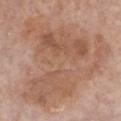Part of a total-body skin-imaging series; this lesion was reviewed on a skin check and was not flagged for biopsy. The lesion is on the front of the torso. A female patient aged 83 or over. Cropped from a total-body skin-imaging series; the visible field is about 15 mm.